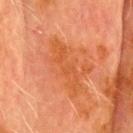Q: Is there a histopathology result?
A: total-body-photography surveillance lesion; no biopsy